A 15 mm close-up tile from a total-body photography series done for melanoma screening; a male patient, approximately 35 years of age; the lesion is located on the back: 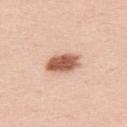Image and clinical context: The lesion's longest dimension is about 4 mm. This is a white-light tile. Diagnosis: Biopsy histopathology demonstrated a benign lesion: dysplastic (Clark) nevus.